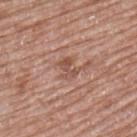Part of a total-body skin-imaging series; this lesion was reviewed on a skin check and was not flagged for biopsy.
This image is a 15 mm lesion crop taken from a total-body photograph.
The recorded lesion diameter is about 2.5 mm.
The lesion is on the left upper arm.
This is a white-light tile.
A female patient roughly 60 years of age.